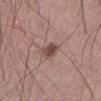Impression:
This lesion was catalogued during total-body skin photography and was not selected for biopsy.
Acquisition and patient details:
The tile uses white-light illumination. The lesion's longest dimension is about 3 mm. A 15 mm close-up tile from a total-body photography series done for melanoma screening. From the left thigh. The patient is a male aged approximately 70. The lesion-visualizer software estimated a border-irregularity index near 2.5/10 and a peripheral color-asymmetry measure near 1.